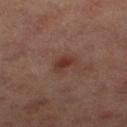follow-up: no biopsy performed (imaged during a skin exam).